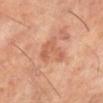– lighting: cross-polarized illumination
– automated metrics: roughly 7 lightness units darker than nearby skin and a normalized border contrast of about 5.5; a border-irregularity index near 6.5/10, a within-lesion color-variation index near 2/10, and a peripheral color-asymmetry measure near 0.5
– location: the right lower leg
– subject: male, aged approximately 60
– size: about 5 mm
– imaging modality: ~15 mm tile from a whole-body skin photo